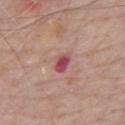The lesion was tiled from a total-body skin photograph and was not biopsied.